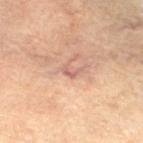Part of a total-body skin-imaging series; this lesion was reviewed on a skin check and was not flagged for biopsy. The subject is a female in their mid-60s. This image is a 15 mm lesion crop taken from a total-body photograph. Located on the left lower leg.